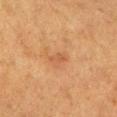Findings:
• patient · female, approximately 40 years of age
• acquisition · ~15 mm tile from a whole-body skin photo
• automated metrics · border irregularity of about 3.5 on a 0–10 scale, a within-lesion color-variation index near 0/10, and a peripheral color-asymmetry measure near 0
• size · about 2.5 mm
• site · the left lower leg
• lighting · cross-polarized illumination